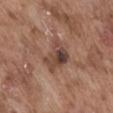biopsy status: imaged on a skin check; not biopsied
subject: male, aged 73 to 77
image: ~15 mm crop, total-body skin-cancer survey
image-analysis metrics: a footprint of about 10 mm², a shape eccentricity near 0.65, and a symmetry-axis asymmetry near 0.35; a lesion color around L≈44 a*≈18 b*≈25 in CIELAB, about 11 CIELAB-L* units darker than the surrounding skin, and a lesion-to-skin contrast of about 8.5 (normalized; higher = more distinct); a color-variation rating of about 10/10 and peripheral color asymmetry of about 3.5; an automated nevus-likeness rating near 0 out of 100
size: ≈4.5 mm
lighting: white-light illumination
location: the abdomen